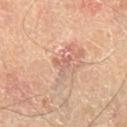No biopsy was performed on this lesion — it was imaged during a full skin examination and was not determined to be concerning.